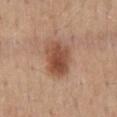follow-up = catalogued during a skin exam; not biopsied | anatomic site = the mid back | acquisition = total-body-photography crop, ~15 mm field of view | lighting = white-light | patient = male, aged approximately 55 | diameter = ~4.5 mm (longest diameter).